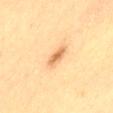The lesion was tiled from a total-body skin photograph and was not biopsied. The tile uses cross-polarized illumination. A female subject aged around 50. Approximately 3.5 mm at its widest. A region of skin cropped from a whole-body photographic capture, roughly 15 mm wide. From the leg.